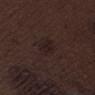Part of a total-body skin-imaging series; this lesion was reviewed on a skin check and was not flagged for biopsy. The subject is a male aged approximately 70. A lesion tile, about 15 mm wide, cut from a 3D total-body photograph. Located on the right thigh.